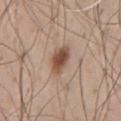Clinical impression:
This lesion was catalogued during total-body skin photography and was not selected for biopsy.
Background:
Captured under white-light illumination. A male subject aged 43–47. The lesion is on the chest. The lesion's longest dimension is about 4 mm. Cropped from a total-body skin-imaging series; the visible field is about 15 mm. The lesion-visualizer software estimated a lesion–skin lightness drop of about 13 and a normalized lesion–skin contrast near 9.5. The analysis additionally found a within-lesion color-variation index near 4.5/10 and peripheral color asymmetry of about 1.5.